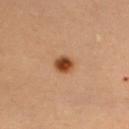workup: total-body-photography surveillance lesion; no biopsy | subject: female, approximately 40 years of age | imaging modality: 15 mm crop, total-body photography | anatomic site: the chest | TBP lesion metrics: a footprint of about 4.5 mm², an outline eccentricity of about 0.6 (0 = round, 1 = elongated), and two-axis asymmetry of about 0.2; a border-irregularity rating of about 1.5/10, internal color variation of about 4 on a 0–10 scale, and a peripheral color-asymmetry measure near 1.5; a lesion-detection confidence of about 100/100 | lesion size: ≈2.5 mm | lighting: cross-polarized illumination.